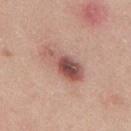notes: total-body-photography surveillance lesion; no biopsy | lesion diameter: about 6 mm | patient: male, aged 23 to 27 | location: the back | tile lighting: white-light | image-analysis metrics: a footprint of about 10 mm², an outline eccentricity of about 0.9 (0 = round, 1 = elongated), and two-axis asymmetry of about 0.4; an automated nevus-likeness rating near 45 out of 100 and a lesion-detection confidence of about 100/100 | image source: ~15 mm tile from a whole-body skin photo.